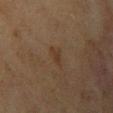Recorded during total-body skin imaging; not selected for excision or biopsy. The recorded lesion diameter is about 2.5 mm. A lesion tile, about 15 mm wide, cut from a 3D total-body photograph. Captured under cross-polarized illumination. A female patient in their mid- to late 50s. The total-body-photography lesion software estimated a footprint of about 3 mm², an eccentricity of roughly 0.8, and a symmetry-axis asymmetry near 0.45. It also reported a border-irregularity index near 4/10 and radial color variation of about 0. On the chest.